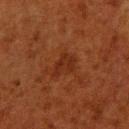Q: Is there a histopathology result?
A: total-body-photography surveillance lesion; no biopsy
Q: What lighting was used for the tile?
A: cross-polarized illumination
Q: What is the imaging modality?
A: ~15 mm crop, total-body skin-cancer survey
Q: What is the lesion's diameter?
A: about 3 mm
Q: What is the anatomic site?
A: the leg
Q: Who is the patient?
A: male, aged approximately 80
Q: Automated lesion metrics?
A: an area of roughly 5 mm² and an outline eccentricity of about 0.7 (0 = round, 1 = elongated); an average lesion color of about L≈23 a*≈22 b*≈27 (CIELAB); border irregularity of about 4.5 on a 0–10 scale, a color-variation rating of about 1.5/10, and radial color variation of about 0.5